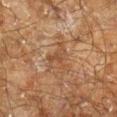Assessment: Part of a total-body skin-imaging series; this lesion was reviewed on a skin check and was not flagged for biopsy. Clinical summary: The lesion's longest dimension is about 4 mm. A roughly 15 mm field-of-view crop from a total-body skin photograph. The total-body-photography lesion software estimated a lesion area of about 7 mm², a shape eccentricity near 0.45, and a symmetry-axis asymmetry near 0.55. The software also gave an average lesion color of about L≈37 a*≈16 b*≈27 (CIELAB), a lesion–skin lightness drop of about 5, and a normalized lesion–skin contrast near 5. And it measured border irregularity of about 6 on a 0–10 scale, a color-variation rating of about 2.5/10, and peripheral color asymmetry of about 1. The analysis additionally found a classifier nevus-likeness of about 0/100 and a detector confidence of about 85 out of 100 that the crop contains a lesion. The subject is a male in their 60s. The lesion is located on the right lower leg. This is a cross-polarized tile.